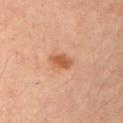Imaged during a routine full-body skin examination; the lesion was not biopsied and no histopathology is available. A region of skin cropped from a whole-body photographic capture, roughly 15 mm wide. Located on the left upper arm. The patient is a male roughly 65 years of age. Imaged with cross-polarized lighting. The recorded lesion diameter is about 2.5 mm. Automated tile analysis of the lesion measured a footprint of about 4.5 mm². The software also gave a nevus-likeness score of about 95/100 and lesion-presence confidence of about 100/100.